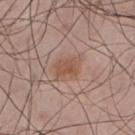Q: Was a biopsy performed?
A: imaged on a skin check; not biopsied
Q: Patient demographics?
A: male, aged 43–47
Q: Lesion location?
A: the upper back
Q: What kind of image is this?
A: 15 mm crop, total-body photography